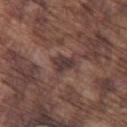Recorded during total-body skin imaging; not selected for excision or biopsy. A male subject, aged around 75. Imaged with white-light lighting. Longest diameter approximately 2.5 mm. The lesion is on the left thigh. This image is a 15 mm lesion crop taken from a total-body photograph.